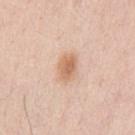<record>
<biopsy_status>not biopsied; imaged during a skin examination</biopsy_status>
<lighting>white-light</lighting>
<site>chest</site>
<image>
  <source>total-body photography crop</source>
  <field_of_view_mm>15</field_of_view_mm>
</image>
<lesion_size>
  <long_diameter_mm_approx>3.0</long_diameter_mm_approx>
</lesion_size>
<patient>
  <sex>male</sex>
  <age_approx>35</age_approx>
</patient>
</record>